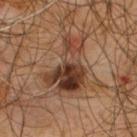Q: Was a biopsy performed?
A: catalogued during a skin exam; not biopsied
Q: Who is the patient?
A: male, about 65 years old
Q: What kind of image is this?
A: ~15 mm crop, total-body skin-cancer survey
Q: Where on the body is the lesion?
A: the upper back